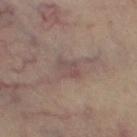Imaged with cross-polarized lighting.
From the right thigh.
The patient is a female approximately 40 years of age.
Cropped from a whole-body photographic skin survey; the tile spans about 15 mm.